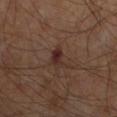Q: Is there a histopathology result?
A: catalogued during a skin exam; not biopsied
Q: Automated lesion metrics?
A: an area of roughly 4 mm², an outline eccentricity of about 0.8 (0 = round, 1 = elongated), and a shape-asymmetry score of about 0.45 (0 = symmetric); a lesion color around L≈27 a*≈18 b*≈20 in CIELAB and a normalized lesion–skin contrast near 8.5; border irregularity of about 4.5 on a 0–10 scale, a within-lesion color-variation index near 4.5/10, and a peripheral color-asymmetry measure near 1.5
Q: How large is the lesion?
A: ≈3 mm
Q: What kind of image is this?
A: 15 mm crop, total-body photography
Q: Where on the body is the lesion?
A: the right forearm
Q: Who is the patient?
A: male, aged 68–72
Q: Illumination type?
A: cross-polarized